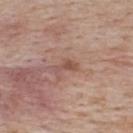Part of a total-body skin-imaging series; this lesion was reviewed on a skin check and was not flagged for biopsy. From the back. A close-up tile cropped from a whole-body skin photograph, about 15 mm across. A male subject approximately 75 years of age.